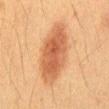Recorded during total-body skin imaging; not selected for excision or biopsy.